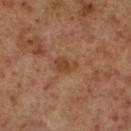* workup — imaged on a skin check; not biopsied
* image source — 15 mm crop, total-body photography
* lighting — cross-polarized
* site — the right lower leg
* lesion diameter — ~3 mm (longest diameter)
* subject — male, approximately 60 years of age